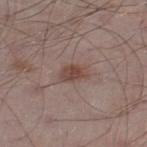notes: no biopsy performed (imaged during a skin exam) | imaging modality: total-body-photography crop, ~15 mm field of view | tile lighting: white-light | lesion diameter: about 3.5 mm | body site: the right thigh | TBP lesion metrics: an average lesion color of about L≈45 a*≈18 b*≈22 (CIELAB), a lesion–skin lightness drop of about 9, and a normalized border contrast of about 7.5; a classifier nevus-likeness of about 75/100 and a detector confidence of about 100 out of 100 that the crop contains a lesion | subject: male, aged 53–57.